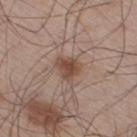Notes:
* follow-up: catalogued during a skin exam; not biopsied
* patient: male, roughly 60 years of age
* body site: the right thigh
* lesion diameter: about 3 mm
* image-analysis metrics: a shape-asymmetry score of about 0.3 (0 = symmetric); a border-irregularity rating of about 3/10, a within-lesion color-variation index near 3.5/10, and peripheral color asymmetry of about 1
* image source: 15 mm crop, total-body photography